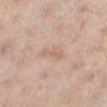This lesion was catalogued during total-body skin photography and was not selected for biopsy.
The lesion-visualizer software estimated a footprint of about 3.5 mm² and a symmetry-axis asymmetry near 0.35. The software also gave border irregularity of about 4 on a 0–10 scale, internal color variation of about 0.5 on a 0–10 scale, and peripheral color asymmetry of about 0.
The lesion's longest dimension is about 3 mm.
The lesion is on the right leg.
A region of skin cropped from a whole-body photographic capture, roughly 15 mm wide.
A female subject, aged around 40.
Captured under cross-polarized illumination.